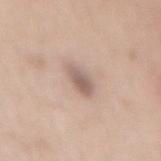The lesion was photographed on a routine skin check and not biopsied; there is no pathology result.
From the mid back.
A close-up tile cropped from a whole-body skin photograph, about 15 mm across.
A female subject about 60 years old.
This is a white-light tile.